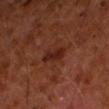workup = no biopsy performed (imaged during a skin exam)
patient = male, aged 58–62
lesion diameter = ~3 mm (longest diameter)
site = the left forearm
image source = ~15 mm tile from a whole-body skin photo
lighting = cross-polarized illumination